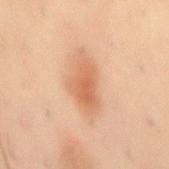Assessment:
Part of a total-body skin-imaging series; this lesion was reviewed on a skin check and was not flagged for biopsy.
Context:
The recorded lesion diameter is about 6.5 mm. A male subject approximately 40 years of age. On the mid back. An algorithmic analysis of the crop reported a lesion area of about 15 mm², an outline eccentricity of about 0.85 (0 = round, 1 = elongated), and two-axis asymmetry of about 0.3. And it measured about 10 CIELAB-L* units darker than the surrounding skin and a lesion-to-skin contrast of about 6.5 (normalized; higher = more distinct). The analysis additionally found radial color variation of about 1. The software also gave a nevus-likeness score of about 80/100. This is a cross-polarized tile. This image is a 15 mm lesion crop taken from a total-body photograph.